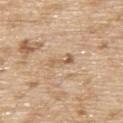biopsy status = imaged on a skin check; not biopsied | lesion diameter = ≈3 mm | image = 15 mm crop, total-body photography | lighting = white-light | subject = male, aged approximately 70 | site = the upper back.